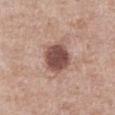Recorded during total-body skin imaging; not selected for excision or biopsy.
A 15 mm close-up tile from a total-body photography series done for melanoma screening.
Located on the chest.
The recorded lesion diameter is about 4.5 mm.
The tile uses white-light illumination.
A male patient aged 68 to 72.